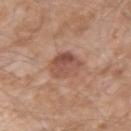Recorded during total-body skin imaging; not selected for excision or biopsy.
A region of skin cropped from a whole-body photographic capture, roughly 15 mm wide.
Located on the arm.
Automated image analysis of the tile measured an average lesion color of about L≈51 a*≈22 b*≈28 (CIELAB), about 10 CIELAB-L* units darker than the surrounding skin, and a normalized lesion–skin contrast near 7. The analysis additionally found a classifier nevus-likeness of about 10/100 and lesion-presence confidence of about 100/100.
Captured under white-light illumination.
The subject is a male aged around 60.